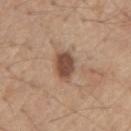Captured during whole-body skin photography for melanoma surveillance; the lesion was not biopsied.
The lesion's longest dimension is about 3 mm.
A region of skin cropped from a whole-body photographic capture, roughly 15 mm wide.
A male patient aged approximately 70.
From the chest.